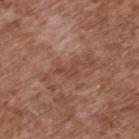The lesion-visualizer software estimated an average lesion color of about L≈45 a*≈22 b*≈28 (CIELAB), a lesion–skin lightness drop of about 6, and a normalized border contrast of about 5. It also reported an automated nevus-likeness rating near 0 out of 100. A 15 mm close-up tile from a total-body photography series done for melanoma screening. Imaged with white-light lighting. From the upper back. A male subject aged approximately 75.